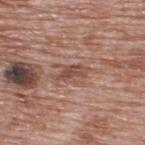Recorded during total-body skin imaging; not selected for excision or biopsy. A 15 mm close-up extracted from a 3D total-body photography capture. A male patient about 70 years old. Captured under white-light illumination. From the upper back.